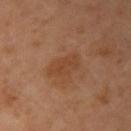Notes:
– workup — imaged on a skin check; not biopsied
– subject — female, approximately 60 years of age
– location — the right upper arm
– imaging modality — total-body-photography crop, ~15 mm field of view
– tile lighting — cross-polarized
– size — ~4 mm (longest diameter)